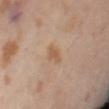Recorded during total-body skin imaging; not selected for excision or biopsy.
A female patient, aged 53–57.
A region of skin cropped from a whole-body photographic capture, roughly 15 mm wide.
Located on the lower back.